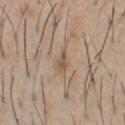Q: Is there a histopathology result?
A: total-body-photography surveillance lesion; no biopsy
Q: What is the lesion's diameter?
A: ~3 mm (longest diameter)
Q: How was the tile lit?
A: white-light illumination
Q: What did automated image analysis measure?
A: a lesion area of about 3.5 mm² and a shape eccentricity near 0.85; roughly 8 lightness units darker than nearby skin and a normalized border contrast of about 6; a classifier nevus-likeness of about 0/100 and a detector confidence of about 80 out of 100 that the crop contains a lesion
Q: What is the imaging modality?
A: 15 mm crop, total-body photography
Q: Where on the body is the lesion?
A: the chest
Q: Who is the patient?
A: male, aged 58–62